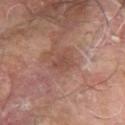notes — imaged on a skin check; not biopsied | lesion diameter — ~2.5 mm (longest diameter) | image source — ~15 mm crop, total-body skin-cancer survey | lighting — cross-polarized illumination | subject — male, aged 63 to 67 | body site — the left forearm.